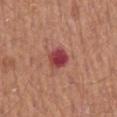Q: Was this lesion biopsied?
A: no biopsy performed (imaged during a skin exam)
Q: Automated lesion metrics?
A: a border-irregularity index near 1.5/10, a color-variation rating of about 5/10, and a peripheral color-asymmetry measure near 1.5; an automated nevus-likeness rating near 0 out of 100 and lesion-presence confidence of about 100/100
Q: What is the imaging modality?
A: total-body-photography crop, ~15 mm field of view
Q: How large is the lesion?
A: ≈3 mm
Q: Lesion location?
A: the mid back
Q: Patient demographics?
A: male, approximately 65 years of age
Q: What lighting was used for the tile?
A: white-light illumination